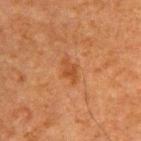notes — total-body-photography surveillance lesion; no biopsy
tile lighting — cross-polarized
location — the upper back
acquisition — 15 mm crop, total-body photography
lesion diameter — ~3 mm (longest diameter)
patient — male, approximately 50 years of age